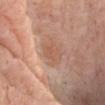Case summary:
* biopsy status — no biopsy performed (imaged during a skin exam)
* size — ~3.5 mm (longest diameter)
* image — ~15 mm tile from a whole-body skin photo
* patient — male, in their mid- to late 70s
* site — the chest
* tile lighting — white-light illumination